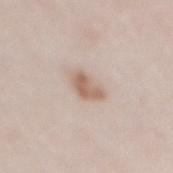{
  "biopsy_status": "not biopsied; imaged during a skin examination",
  "image": {
    "source": "total-body photography crop",
    "field_of_view_mm": 15
  },
  "patient": {
    "sex": "female",
    "age_approx": 40
  },
  "automated_metrics": {
    "area_mm2_approx": 6.0,
    "shape_asymmetry": 0.35,
    "border_irregularity_0_10": 3.5,
    "color_variation_0_10": 3.5,
    "peripheral_color_asymmetry": 1.0
  },
  "site": "back"
}